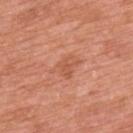Part of a total-body skin-imaging series; this lesion was reviewed on a skin check and was not flagged for biopsy. Imaged with white-light lighting. A male subject, in their mid- to late 60s. This image is a 15 mm lesion crop taken from a total-body photograph. Located on the upper back.